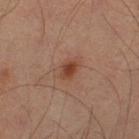biopsy status: catalogued during a skin exam; not biopsied
acquisition: ~15 mm tile from a whole-body skin photo
diameter: ~2.5 mm (longest diameter)
lighting: cross-polarized
site: the left thigh
subject: male, approximately 60 years of age